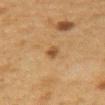biopsy status = imaged on a skin check; not biopsied
lighting = cross-polarized
subject = female, roughly 45 years of age
image source = ~15 mm crop, total-body skin-cancer survey
body site = the right upper arm